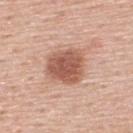The lesion was tiled from a total-body skin photograph and was not biopsied.